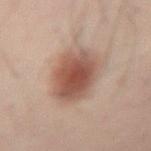Clinical impression: This lesion was catalogued during total-body skin photography and was not selected for biopsy. Context: From the right upper arm. The patient is a male aged around 50. A roughly 15 mm field-of-view crop from a total-body skin photograph. The lesion's longest dimension is about 6 mm. This is a cross-polarized tile.